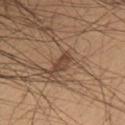Impression:
Part of a total-body skin-imaging series; this lesion was reviewed on a skin check and was not flagged for biopsy.
Background:
Captured under white-light illumination. The subject is a male in their mid- to late 50s. Cropped from a total-body skin-imaging series; the visible field is about 15 mm. The lesion is on the chest. Approximately 3 mm at its widest. The total-body-photography lesion software estimated border irregularity of about 3 on a 0–10 scale and a within-lesion color-variation index near 1/10. The software also gave a nevus-likeness score of about 5/100.